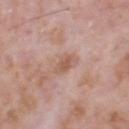Clinical impression:
Recorded during total-body skin imaging; not selected for excision or biopsy.
Acquisition and patient details:
The patient is a male roughly 70 years of age. On the head or neck. Cropped from a total-body skin-imaging series; the visible field is about 15 mm.